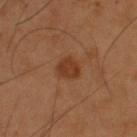biopsy status=catalogued during a skin exam; not biopsied
anatomic site=the back
subject=male, aged 53–57
lesion diameter=about 2.5 mm
image source=total-body-photography crop, ~15 mm field of view